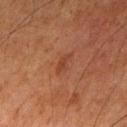Impression:
Captured during whole-body skin photography for melanoma surveillance; the lesion was not biopsied.
Acquisition and patient details:
The subject is a male aged 78 to 82. From the leg. The lesion's longest dimension is about 2.5 mm. Cropped from a whole-body photographic skin survey; the tile spans about 15 mm. The total-body-photography lesion software estimated a lesion–skin lightness drop of about 5 and a lesion-to-skin contrast of about 5 (normalized; higher = more distinct). It also reported border irregularity of about 3 on a 0–10 scale and a within-lesion color-variation index near 1.5/10. It also reported a classifier nevus-likeness of about 5/100 and lesion-presence confidence of about 100/100. Captured under cross-polarized illumination.